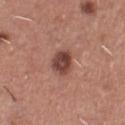<lesion>
  <biopsy_status>not biopsied; imaged during a skin examination</biopsy_status>
  <patient>
    <sex>male</sex>
    <age_approx>45</age_approx>
  </patient>
  <image>
    <source>total-body photography crop</source>
    <field_of_view_mm>15</field_of_view_mm>
  </image>
  <site>chest</site>
</lesion>